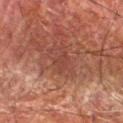biopsy status = no biopsy performed (imaged during a skin exam) | size = ~3 mm (longest diameter) | location = the right forearm | subject = male, aged 78 to 82 | image = 15 mm crop, total-body photography.